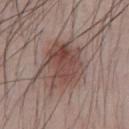Part of a total-body skin-imaging series; this lesion was reviewed on a skin check and was not flagged for biopsy. The lesion is located on the abdomen. A male patient aged 38 to 42. Imaged with white-light lighting. A 15 mm close-up extracted from a 3D total-body photography capture.